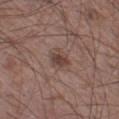Impression: The lesion was tiled from a total-body skin photograph and was not biopsied. Background: A male patient aged approximately 50. The recorded lesion diameter is about 3 mm. The total-body-photography lesion software estimated a lesion color around L≈42 a*≈18 b*≈21 in CIELAB and roughly 9 lightness units darker than nearby skin. It also reported a border-irregularity rating of about 2.5/10 and peripheral color asymmetry of about 1. On the left thigh. The tile uses white-light illumination. A 15 mm close-up tile from a total-body photography series done for melanoma screening.